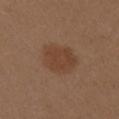Findings:
– workup: no biopsy performed (imaged during a skin exam)
– image source: 15 mm crop, total-body photography
– body site: the right upper arm
– size: about 5 mm
– subject: female, about 40 years old
– TBP lesion metrics: an eccentricity of roughly 0.65 and a symmetry-axis asymmetry near 0.15